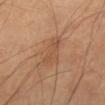The lesion was photographed on a routine skin check and not biopsied; there is no pathology result.
Located on the left lower leg.
A male patient, aged approximately 70.
Approximately 4 mm at its widest.
A 15 mm crop from a total-body photograph taken for skin-cancer surveillance.